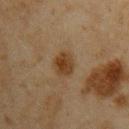site: the right upper arm | lesion size: about 3 mm | illumination: cross-polarized | imaging modality: total-body-photography crop, ~15 mm field of view | image-analysis metrics: a mean CIELAB color near L≈32 a*≈14 b*≈28 and roughly 8 lightness units darker than nearby skin; a border-irregularity index near 2/10, a color-variation rating of about 3.5/10, and radial color variation of about 1 | subject: male, aged around 45.